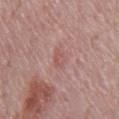Imaged during a routine full-body skin examination; the lesion was not biopsied and no histopathology is available.
A region of skin cropped from a whole-body photographic capture, roughly 15 mm wide.
A male patient, approximately 65 years of age.
The total-body-photography lesion software estimated a footprint of about 3 mm². The analysis additionally found a lesion color around L≈54 a*≈23 b*≈23 in CIELAB and a lesion-to-skin contrast of about 4.5 (normalized; higher = more distinct). The analysis additionally found a border-irregularity rating of about 2.5/10, a within-lesion color-variation index near 1/10, and a peripheral color-asymmetry measure near 0.5. And it measured an automated nevus-likeness rating near 0 out of 100 and a detector confidence of about 100 out of 100 that the crop contains a lesion.
The lesion's longest dimension is about 2.5 mm.
Located on the chest.
This is a white-light tile.